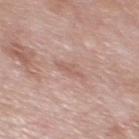  biopsy_status: not biopsied; imaged during a skin examination
  image:
    source: total-body photography crop
    field_of_view_mm: 15
  patient:
    sex: male
    age_approx: 55
  site: chest